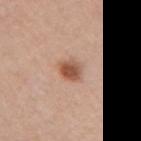Findings:
– notes: imaged on a skin check; not biopsied
– imaging modality: ~15 mm crop, total-body skin-cancer survey
– patient: female, aged 58 to 62
– size: ≈3 mm
– image-analysis metrics: a lesion area of about 5.5 mm², a shape eccentricity near 0.55, and a symmetry-axis asymmetry near 0.2
– body site: the right upper arm